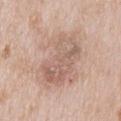The lesion was tiled from a total-body skin photograph and was not biopsied. A 15 mm close-up extracted from a 3D total-body photography capture. The lesion is located on the back. The subject is a male roughly 65 years of age.